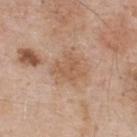No biopsy was performed on this lesion — it was imaged during a full skin examination and was not determined to be concerning.
A close-up tile cropped from a whole-body skin photograph, about 15 mm across.
The patient is a male aged 58 to 62.
The lesion is located on the upper back.